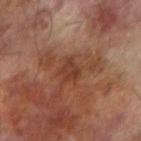Q: Was a biopsy performed?
A: catalogued during a skin exam; not biopsied
Q: What kind of image is this?
A: total-body-photography crop, ~15 mm field of view
Q: How large is the lesion?
A: ~2.5 mm (longest diameter)
Q: What are the patient's age and sex?
A: male, about 65 years old
Q: Automated lesion metrics?
A: a footprint of about 4 mm², an outline eccentricity of about 0.7 (0 = round, 1 = elongated), and a symmetry-axis asymmetry near 0.3; roughly 7 lightness units darker than nearby skin and a normalized border contrast of about 6.5
Q: Lesion location?
A: the right forearm
Q: Illumination type?
A: cross-polarized